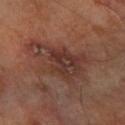| field | value |
|---|---|
| biopsy status | total-body-photography surveillance lesion; no biopsy |